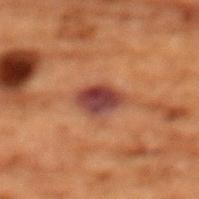workup: total-body-photography surveillance lesion; no biopsy | automated metrics: a footprint of about 7.5 mm² and a shape-asymmetry score of about 0.15 (0 = symmetric); a lesion color around L≈31 a*≈22 b*≈21 in CIELAB, about 12 CIELAB-L* units darker than the surrounding skin, and a normalized lesion–skin contrast near 13; a border-irregularity rating of about 2/10, a within-lesion color-variation index near 4/10, and peripheral color asymmetry of about 1; a nevus-likeness score of about 90/100 | illumination: cross-polarized | body site: the chest | subject: female, aged around 80 | imaging modality: ~15 mm tile from a whole-body skin photo | lesion diameter: ≈3.5 mm.